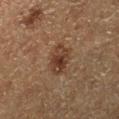Part of a total-body skin-imaging series; this lesion was reviewed on a skin check and was not flagged for biopsy.
This is a cross-polarized tile.
Located on the right lower leg.
A male patient roughly 75 years of age.
The lesion's longest dimension is about 3.5 mm.
The total-body-photography lesion software estimated a lesion area of about 7.5 mm² and a symmetry-axis asymmetry near 0.2. It also reported a lesion–skin lightness drop of about 8 and a normalized border contrast of about 8. And it measured border irregularity of about 2 on a 0–10 scale, internal color variation of about 4 on a 0–10 scale, and a peripheral color-asymmetry measure near 1.5. And it measured a classifier nevus-likeness of about 85/100 and a lesion-detection confidence of about 100/100.
Cropped from a total-body skin-imaging series; the visible field is about 15 mm.